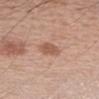{
  "biopsy_status": "not biopsied; imaged during a skin examination",
  "automated_metrics": {
    "border_irregularity_0_10": 2.0,
    "peripheral_color_asymmetry": 0.5
  },
  "lesion_size": {
    "long_diameter_mm_approx": 2.5
  },
  "site": "arm",
  "image": {
    "source": "total-body photography crop",
    "field_of_view_mm": 15
  },
  "patient": {
    "sex": "male",
    "age_approx": 60
  },
  "lighting": "white-light"
}